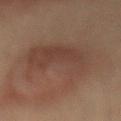Recorded during total-body skin imaging; not selected for excision or biopsy. A male subject aged around 55. Imaged with cross-polarized lighting. Located on the lower back. The recorded lesion diameter is about 10.5 mm. The lesion-visualizer software estimated a lesion area of about 37 mm², an outline eccentricity of about 0.75 (0 = round, 1 = elongated), and a shape-asymmetry score of about 0.35 (0 = symmetric). The analysis additionally found a lesion color around L≈33 a*≈14 b*≈21 in CIELAB and roughly 6 lightness units darker than nearby skin. It also reported a border-irregularity rating of about 6.5/10 and a within-lesion color-variation index near 3/10. The software also gave a classifier nevus-likeness of about 20/100 and a detector confidence of about 100 out of 100 that the crop contains a lesion. A 15 mm crop from a total-body photograph taken for skin-cancer surveillance.